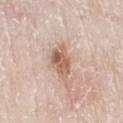follow-up = catalogued during a skin exam; not biopsied | patient = male, aged 78–82 | image-analysis metrics = a footprint of about 8.5 mm² | image = ~15 mm crop, total-body skin-cancer survey | location = the right lower leg.